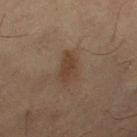{
  "biopsy_status": "not biopsied; imaged during a skin examination",
  "site": "right thigh",
  "image": {
    "source": "total-body photography crop",
    "field_of_view_mm": 15
  },
  "lesion_size": {
    "long_diameter_mm_approx": 4.0
  },
  "patient": {
    "sex": "female",
    "age_approx": 55
  }
}